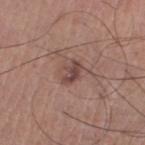No biopsy was performed on this lesion — it was imaged during a full skin examination and was not determined to be concerning. The patient is a male aged around 55. On the left thigh. The recorded lesion diameter is about 3 mm. The lesion-visualizer software estimated an area of roughly 4 mm², an outline eccentricity of about 0.8 (0 = round, 1 = elongated), and a shape-asymmetry score of about 0.3 (0 = symmetric). And it measured border irregularity of about 3.5 on a 0–10 scale, internal color variation of about 2 on a 0–10 scale, and peripheral color asymmetry of about 0.5. The analysis additionally found a classifier nevus-likeness of about 30/100 and a detector confidence of about 100 out of 100 that the crop contains a lesion. This is a white-light tile. Cropped from a whole-body photographic skin survey; the tile spans about 15 mm.